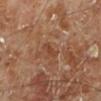Case summary:
* subject · male, aged 68–72
* imaging modality · ~15 mm tile from a whole-body skin photo
* body site · the leg